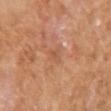biopsy_status: not biopsied; imaged during a skin examination
image:
  source: total-body photography crop
  field_of_view_mm: 15
site: left upper arm
lesion_size:
  long_diameter_mm_approx: 2.5
lighting: white-light
patient:
  sex: male
  age_approx: 75